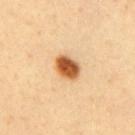The lesion was photographed on a routine skin check and not biopsied; there is no pathology result.